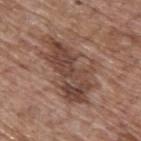{"biopsy_status": "not biopsied; imaged during a skin examination", "patient": {"sex": "male", "age_approx": 70}, "lighting": "white-light", "image": {"source": "total-body photography crop", "field_of_view_mm": 15}, "site": "upper back"}